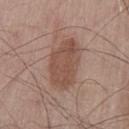Recorded during total-body skin imaging; not selected for excision or biopsy.
Measured at roughly 6.5 mm in maximum diameter.
The lesion is located on the left thigh.
A 15 mm close-up tile from a total-body photography series done for melanoma screening.
The patient is a male aged around 55.
Captured under white-light illumination.